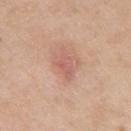Impression: Recorded during total-body skin imaging; not selected for excision or biopsy. Image and clinical context: Approximately 3 mm at its widest. This is a white-light tile. A roughly 15 mm field-of-view crop from a total-body skin photograph. The patient is a female roughly 40 years of age. An algorithmic analysis of the crop reported a lesion area of about 4 mm², an outline eccentricity of about 0.8 (0 = round, 1 = elongated), and two-axis asymmetry of about 0.35. It also reported an average lesion color of about L≈59 a*≈25 b*≈27 (CIELAB), about 8 CIELAB-L* units darker than the surrounding skin, and a normalized border contrast of about 5.5. It also reported a lesion-detection confidence of about 100/100. On the left upper arm.